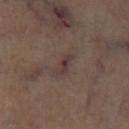{
  "biopsy_status": "not biopsied; imaged during a skin examination",
  "image": {
    "source": "total-body photography crop",
    "field_of_view_mm": 15
  },
  "lighting": "cross-polarized",
  "site": "left lower leg",
  "lesion_size": {
    "long_diameter_mm_approx": 3.0
  }
}